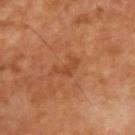This lesion was catalogued during total-body skin photography and was not selected for biopsy.
A male patient, approximately 55 years of age.
The lesion is on the chest.
Captured under cross-polarized illumination.
Approximately 3.5 mm at its widest.
The lesion-visualizer software estimated a mean CIELAB color near L≈43 a*≈25 b*≈36, a lesion–skin lightness drop of about 6, and a normalized lesion–skin contrast near 5. The analysis additionally found a border-irregularity index near 7.5/10, internal color variation of about 0 on a 0–10 scale, and a peripheral color-asymmetry measure near 0. The software also gave a classifier nevus-likeness of about 0/100 and a detector confidence of about 100 out of 100 that the crop contains a lesion.
Cropped from a total-body skin-imaging series; the visible field is about 15 mm.